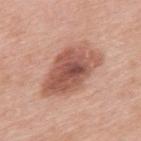image:
  source: total-body photography crop
  field_of_view_mm: 15
lesion_size:
  long_diameter_mm_approx: 8.0
site: upper back
patient:
  sex: female
  age_approx: 60